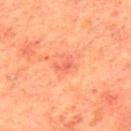Assessment:
Part of a total-body skin-imaging series; this lesion was reviewed on a skin check and was not flagged for biopsy.
Image and clinical context:
A 15 mm close-up extracted from a 3D total-body photography capture. A male patient about 65 years old. The lesion-visualizer software estimated border irregularity of about 6 on a 0–10 scale, internal color variation of about 0 on a 0–10 scale, and radial color variation of about 0. And it measured a classifier nevus-likeness of about 5/100. The lesion is on the mid back. The tile uses cross-polarized illumination.